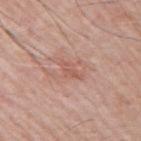This lesion was catalogued during total-body skin photography and was not selected for biopsy.
A region of skin cropped from a whole-body photographic capture, roughly 15 mm wide.
The patient is a male in their mid-60s.
This is a white-light tile.
The recorded lesion diameter is about 3 mm.
The total-body-photography lesion software estimated a footprint of about 3 mm², an outline eccentricity of about 0.9 (0 = round, 1 = elongated), and two-axis asymmetry of about 0.35. And it measured a lesion color around L≈57 a*≈24 b*≈27 in CIELAB and a lesion–skin lightness drop of about 7. And it measured a classifier nevus-likeness of about 0/100 and a detector confidence of about 100 out of 100 that the crop contains a lesion.
Located on the right upper arm.